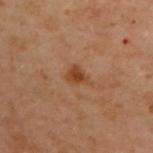Cropped from a whole-body photographic skin survey; the tile spans about 15 mm. Automated tile analysis of the lesion measured an average lesion color of about L≈38 a*≈20 b*≈32 (CIELAB), a lesion–skin lightness drop of about 8, and a lesion-to-skin contrast of about 7.5 (normalized; higher = more distinct). The software also gave a border-irregularity index near 3/10, a within-lesion color-variation index near 2/10, and radial color variation of about 0.5. The analysis additionally found an automated nevus-likeness rating near 80 out of 100 and a lesion-detection confidence of about 100/100. The patient is a female approximately 60 years of age. The lesion's longest dimension is about 2.5 mm. Located on the upper back.